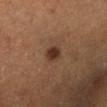Q: Was a biopsy performed?
A: total-body-photography surveillance lesion; no biopsy
Q: Where on the body is the lesion?
A: the leg
Q: How was the tile lit?
A: cross-polarized illumination
Q: What are the patient's age and sex?
A: male, about 40 years old
Q: Lesion size?
A: ≈2 mm
Q: What is the imaging modality?
A: ~15 mm crop, total-body skin-cancer survey